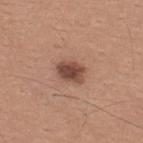Q: Was a biopsy performed?
A: imaged on a skin check; not biopsied
Q: How large is the lesion?
A: ≈3.5 mm
Q: What is the anatomic site?
A: the upper back
Q: Patient demographics?
A: male, about 30 years old
Q: Automated lesion metrics?
A: a mean CIELAB color near L≈47 a*≈21 b*≈26, a lesion–skin lightness drop of about 14, and a lesion-to-skin contrast of about 10 (normalized; higher = more distinct); border irregularity of about 2 on a 0–10 scale and internal color variation of about 4 on a 0–10 scale
Q: How was this image acquired?
A: ~15 mm crop, total-body skin-cancer survey